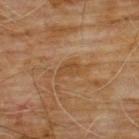Q: Is there a histopathology result?
A: catalogued during a skin exam; not biopsied
Q: What is the imaging modality?
A: ~15 mm crop, total-body skin-cancer survey
Q: How large is the lesion?
A: about 2.5 mm
Q: Where on the body is the lesion?
A: the chest
Q: What are the patient's age and sex?
A: male, aged approximately 60
Q: How was the tile lit?
A: cross-polarized